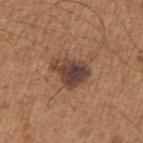Case summary:
* notes · total-body-photography surveillance lesion; no biopsy
* acquisition · total-body-photography crop, ~15 mm field of view
* patient · male, approximately 65 years of age
* lesion diameter · about 4 mm
* body site · the right upper arm